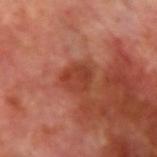<lesion>
<biopsy_status>not biopsied; imaged during a skin examination</biopsy_status>
<site>right upper arm</site>
<image>
  <source>total-body photography crop</source>
  <field_of_view_mm>15</field_of_view_mm>
</image>
<lighting>cross-polarized</lighting>
<patient>
  <sex>male</sex>
  <age_approx>70</age_approx>
</patient>
</lesion>